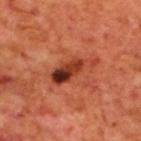Q: Was a biopsy performed?
A: total-body-photography surveillance lesion; no biopsy
Q: How large is the lesion?
A: about 5.5 mm
Q: What did automated image analysis measure?
A: a mean CIELAB color near L≈38 a*≈31 b*≈34, about 14 CIELAB-L* units darker than the surrounding skin, and a normalized border contrast of about 10.5; a border-irregularity index near 4.5/10, internal color variation of about 10 on a 0–10 scale, and peripheral color asymmetry of about 3; a classifier nevus-likeness of about 15/100 and a lesion-detection confidence of about 100/100
Q: What are the patient's age and sex?
A: male, about 70 years old
Q: How was this image acquired?
A: ~15 mm tile from a whole-body skin photo
Q: Where on the body is the lesion?
A: the upper back